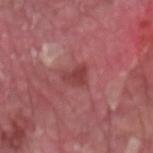Captured during whole-body skin photography for melanoma surveillance; the lesion was not biopsied. On the left forearm. This is a white-light tile. A male patient, aged approximately 40. This image is a 15 mm lesion crop taken from a total-body photograph. Approximately 2.5 mm at its widest.